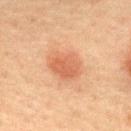Notes:
– patient — male, aged around 60
– tile lighting — cross-polarized illumination
– image — total-body-photography crop, ~15 mm field of view
– body site — the mid back
– lesion size — about 4 mm